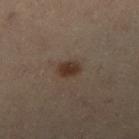No biopsy was performed on this lesion — it was imaged during a full skin examination and was not determined to be concerning. An algorithmic analysis of the crop reported a nevus-likeness score of about 95/100 and lesion-presence confidence of about 100/100. Longest diameter approximately 2.5 mm. A region of skin cropped from a whole-body photographic capture, roughly 15 mm wide. Located on the right lower leg. A male patient aged 53–57.